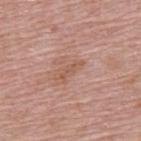Imaged during a routine full-body skin examination; the lesion was not biopsied and no histopathology is available. Cropped from a whole-body photographic skin survey; the tile spans about 15 mm. Located on the upper back. The lesion-visualizer software estimated an area of roughly 2.5 mm² and two-axis asymmetry of about 0.4. The analysis additionally found a border-irregularity index near 5.5/10. It also reported an automated nevus-likeness rating near 0 out of 100 and a detector confidence of about 100 out of 100 that the crop contains a lesion. This is a white-light tile. The subject is a male aged 68–72. Longest diameter approximately 3 mm.